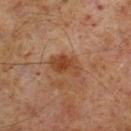– workup: catalogued during a skin exam; not biopsied
– body site: the right lower leg
– image source: 15 mm crop, total-body photography
– size: ≈4 mm
– subject: male, about 60 years old
– illumination: cross-polarized illumination
– image-analysis metrics: an area of roughly 7.5 mm², a shape eccentricity near 0.85, and two-axis asymmetry of about 0.25; a detector confidence of about 100 out of 100 that the crop contains a lesion